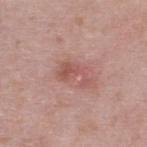This lesion was catalogued during total-body skin photography and was not selected for biopsy.
A roughly 15 mm field-of-view crop from a total-body skin photograph.
Measured at roughly 4 mm in maximum diameter.
The subject is a male aged 38–42.
The lesion is located on the back.